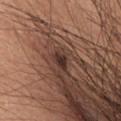| feature | finding |
|---|---|
| follow-up | catalogued during a skin exam; not biopsied |
| anatomic site | the chest |
| illumination | white-light illumination |
| subject | male, aged 58 to 62 |
| image | ~15 mm crop, total-body skin-cancer survey |